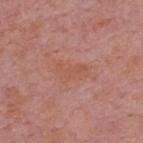The lesion was tiled from a total-body skin photograph and was not biopsied.
The lesion-visualizer software estimated an area of roughly 5.5 mm². And it measured roughly 5 lightness units darker than nearby skin and a normalized border contrast of about 5. The software also gave border irregularity of about 4.5 on a 0–10 scale, a within-lesion color-variation index near 1.5/10, and a peripheral color-asymmetry measure near 0.5. The analysis additionally found a nevus-likeness score of about 0/100 and a detector confidence of about 100 out of 100 that the crop contains a lesion.
Captured under white-light illumination.
Cropped from a total-body skin-imaging series; the visible field is about 15 mm.
Located on the upper back.
A male patient aged around 75.
Measured at roughly 3.5 mm in maximum diameter.